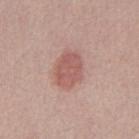patient: male, roughly 40 years of age; imaging modality: 15 mm crop, total-body photography; body site: the abdomen.